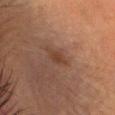| feature | finding |
|---|---|
| notes | no biopsy performed (imaged during a skin exam) |